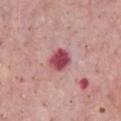biopsy_status: not biopsied; imaged during a skin examination
automated_metrics:
  area_mm2_approx: 6.0
  eccentricity: 0.5
  shape_asymmetry: 0.15
  cielab_L: 48
  cielab_a: 35
  cielab_b: 18
  vs_skin_darker_L: 17.0
  border_irregularity_0_10: 1.5
  color_variation_0_10: 3.5
  peripheral_color_asymmetry: 1.0
  nevus_likeness_0_100: 0
site: chest
patient:
  sex: male
  age_approx: 65
lesion_size:
  long_diameter_mm_approx: 3.0
image:
  source: total-body photography crop
  field_of_view_mm: 15